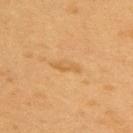<case>
<biopsy_status>not biopsied; imaged during a skin examination</biopsy_status>
<patient>
  <sex>male</sex>
  <age_approx>55</age_approx>
</patient>
<lesion_size>
  <long_diameter_mm_approx>3.5</long_diameter_mm_approx>
</lesion_size>
<image>
  <source>total-body photography crop</source>
  <field_of_view_mm>15</field_of_view_mm>
</image>
<lighting>cross-polarized</lighting>
<automated_metrics>
  <area_mm2_approx>4.0</area_mm2_approx>
  <eccentricity>0.95</eccentricity>
  <shape_asymmetry>0.25</shape_asymmetry>
  <cielab_L>55</cielab_L>
  <cielab_a>19</cielab_a>
  <cielab_b>40</cielab_b>
  <vs_skin_darker_L>6.0</vs_skin_darker_L>
  <vs_skin_contrast_norm>5.0</vs_skin_contrast_norm>
  <border_irregularity_0_10>3.0</border_irregularity_0_10>
  <color_variation_0_10>1.0</color_variation_0_10>
  <peripheral_color_asymmetry>0.5</peripheral_color_asymmetry>
  <lesion_detection_confidence_0_100>95</lesion_detection_confidence_0_100>
</automated_metrics>
<site>upper back</site>
</case>